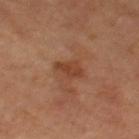Clinical impression: Imaged during a routine full-body skin examination; the lesion was not biopsied and no histopathology is available. Clinical summary: On the right thigh. A female subject, roughly 65 years of age. The total-body-photography lesion software estimated an average lesion color of about L≈41 a*≈23 b*≈32 (CIELAB) and a normalized border contrast of about 6.5. And it measured a border-irregularity index near 2/10 and a color-variation rating of about 2/10. And it measured a detector confidence of about 100 out of 100 that the crop contains a lesion. Cropped from a whole-body photographic skin survey; the tile spans about 15 mm. Imaged with cross-polarized lighting. About 3 mm across.